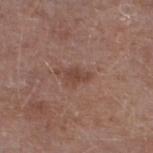Captured during whole-body skin photography for melanoma surveillance; the lesion was not biopsied.
From the left lower leg.
The subject is a male in their mid-60s.
A close-up tile cropped from a whole-body skin photograph, about 15 mm across.